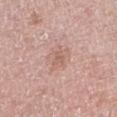Part of a total-body skin-imaging series; this lesion was reviewed on a skin check and was not flagged for biopsy. The subject is a female aged approximately 65. From the right upper arm. A region of skin cropped from a whole-body photographic capture, roughly 15 mm wide.